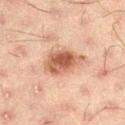The lesion was tiled from a total-body skin photograph and was not biopsied. The lesion is on the left thigh. The subject is a male aged 48 to 52. A lesion tile, about 15 mm wide, cut from a 3D total-body photograph.